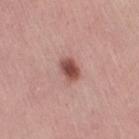Captured during whole-body skin photography for melanoma surveillance; the lesion was not biopsied.
An algorithmic analysis of the crop reported border irregularity of about 1.5 on a 0–10 scale, internal color variation of about 3.5 on a 0–10 scale, and radial color variation of about 1. And it measured an automated nevus-likeness rating near 100 out of 100 and a lesion-detection confidence of about 100/100.
The lesion is located on the leg.
A female patient in their 50s.
About 2.5 mm across.
Cropped from a whole-body photographic skin survey; the tile spans about 15 mm.
Imaged with white-light lighting.